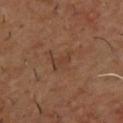Recorded during total-body skin imaging; not selected for excision or biopsy.
The lesion's longest dimension is about 3 mm.
From the upper back.
A close-up tile cropped from a whole-body skin photograph, about 15 mm across.
The patient is a male aged around 55.
Captured under cross-polarized illumination.